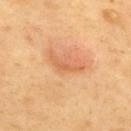follow-up: catalogued during a skin exam; not biopsied | patient: female, about 60 years old | location: the back | illumination: cross-polarized | lesion size: ~4 mm (longest diameter) | image source: total-body-photography crop, ~15 mm field of view | automated metrics: an average lesion color of about L≈65 a*≈27 b*≈42 (CIELAB), roughly 9 lightness units darker than nearby skin, and a normalized border contrast of about 5.5.